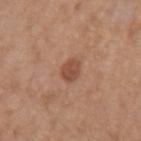{
  "biopsy_status": "not biopsied; imaged during a skin examination",
  "lesion_size": {
    "long_diameter_mm_approx": 2.5
  },
  "lighting": "white-light",
  "image": {
    "source": "total-body photography crop",
    "field_of_view_mm": 15
  },
  "site": "arm",
  "patient": {
    "sex": "female",
    "age_approx": 60
  }
}